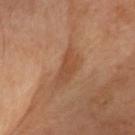Imaged during a routine full-body skin examination; the lesion was not biopsied and no histopathology is available.
An algorithmic analysis of the crop reported an average lesion color of about L≈41 a*≈20 b*≈30 (CIELAB), about 7 CIELAB-L* units darker than the surrounding skin, and a normalized border contrast of about 6. And it measured a border-irregularity index near 3.5/10, internal color variation of about 2 on a 0–10 scale, and radial color variation of about 1.
The lesion is on the head or neck.
Cropped from a total-body skin-imaging series; the visible field is about 15 mm.
Captured under cross-polarized illumination.
The patient is a female aged around 60.
Approximately 4 mm at its widest.